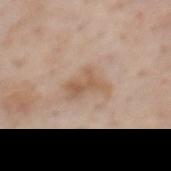automated lesion analysis: a footprint of about 6.5 mm², an eccentricity of roughly 0.8, and a shape-asymmetry score of about 0.4 (0 = symmetric); a border-irregularity rating of about 5.5/10, a within-lesion color-variation index near 2.5/10, and a peripheral color-asymmetry measure near 1
image: ~15 mm tile from a whole-body skin photo
anatomic site: the mid back
subject: male, approximately 60 years of age